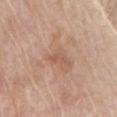notes: no biopsy performed (imaged during a skin exam) | site: the chest | subject: male, approximately 65 years of age | imaging modality: total-body-photography crop, ~15 mm field of view | TBP lesion metrics: a lesion color around L≈56 a*≈21 b*≈31 in CIELAB, roughly 7 lightness units darker than nearby skin, and a lesion-to-skin contrast of about 5 (normalized; higher = more distinct); a border-irregularity rating of about 5.5/10 and a color-variation rating of about 0/10; an automated nevus-likeness rating near 0 out of 100 and lesion-presence confidence of about 100/100.